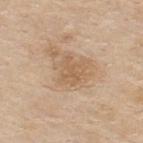notes: total-body-photography surveillance lesion; no biopsy | illumination: white-light | body site: the upper back | acquisition: 15 mm crop, total-body photography | automated lesion analysis: an area of roughly 5 mm², an outline eccentricity of about 0.65 (0 = round, 1 = elongated), and a symmetry-axis asymmetry near 0.3; an average lesion color of about L≈59 a*≈17 b*≈33 (CIELAB), roughly 7 lightness units darker than nearby skin, and a normalized lesion–skin contrast near 5.5; a lesion-detection confidence of about 100/100 | subject: male, about 80 years old | lesion size: about 3 mm.